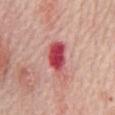This lesion was catalogued during total-body skin photography and was not selected for biopsy.
This is a white-light tile.
About 4.5 mm across.
The patient is a female in their mid- to late 60s.
This image is a 15 mm lesion crop taken from a total-body photograph.
The lesion is on the front of the torso.